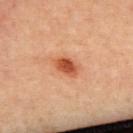About 3 mm across.
A lesion tile, about 15 mm wide, cut from a 3D total-body photograph.
Captured under cross-polarized illumination.
The subject is a female approximately 45 years of age.
Automated image analysis of the tile measured a shape eccentricity near 0.75 and a shape-asymmetry score of about 0.15 (0 = symmetric). The software also gave an average lesion color of about L≈54 a*≈31 b*≈38 (CIELAB) and a lesion–skin lightness drop of about 14. And it measured a border-irregularity index near 1.5/10 and radial color variation of about 1. The analysis additionally found a nevus-likeness score of about 100/100 and a detector confidence of about 100 out of 100 that the crop contains a lesion.
On the upper back.